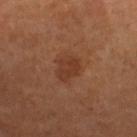The lesion was photographed on a routine skin check and not biopsied; there is no pathology result. The tile uses cross-polarized illumination. Longest diameter approximately 3 mm. A roughly 15 mm field-of-view crop from a total-body skin photograph. On the leg. The patient is a female in their mid-60s.